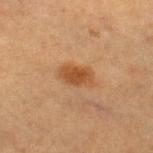Imaged during a routine full-body skin examination; the lesion was not biopsied and no histopathology is available.
Captured under cross-polarized illumination.
A 15 mm close-up tile from a total-body photography series done for melanoma screening.
The lesion-visualizer software estimated a footprint of about 6.5 mm² and an eccentricity of roughly 0.85. It also reported an automated nevus-likeness rating near 95 out of 100 and a lesion-detection confidence of about 100/100.
About 4 mm across.
The subject is a female aged 38–42.
From the right lower leg.